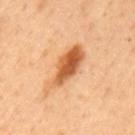biopsy status: total-body-photography surveillance lesion; no biopsy
site: the mid back
image source: 15 mm crop, total-body photography
subject: male, aged approximately 50